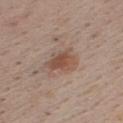The lesion was photographed on a routine skin check and not biopsied; there is no pathology result.
The tile uses white-light illumination.
A male subject about 45 years old.
A 15 mm crop from a total-body photograph taken for skin-cancer surveillance.
On the chest.
About 3.5 mm across.